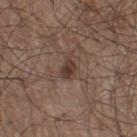Recorded during total-body skin imaging; not selected for excision or biopsy. Located on the left thigh. This is a white-light tile. A male patient, approximately 75 years of age. Approximately 3 mm at its widest. A region of skin cropped from a whole-body photographic capture, roughly 15 mm wide.